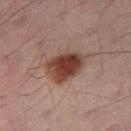Part of a total-body skin-imaging series; this lesion was reviewed on a skin check and was not flagged for biopsy.
Cropped from a whole-body photographic skin survey; the tile spans about 15 mm.
On the right thigh.
A male subject, roughly 50 years of age.